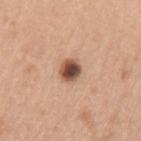<case>
<biopsy_status>not biopsied; imaged during a skin examination</biopsy_status>
<patient>
  <sex>female</sex>
  <age_approx>55</age_approx>
</patient>
<lesion_size>
  <long_diameter_mm_approx>2.5</long_diameter_mm_approx>
</lesion_size>
<image>
  <source>total-body photography crop</source>
  <field_of_view_mm>15</field_of_view_mm>
</image>
<lighting>white-light</lighting>
<site>arm</site>
</case>